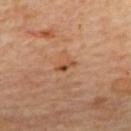biopsy status = catalogued during a skin exam; not biopsied.